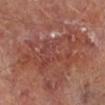Impression: Captured during whole-body skin photography for melanoma surveillance; the lesion was not biopsied. Image and clinical context: About 11 mm across. Cropped from a whole-body photographic skin survey; the tile spans about 15 mm. A male patient aged around 65. The lesion is located on the left lower leg. The total-body-photography lesion software estimated an area of roughly 32 mm², an eccentricity of roughly 0.9, and a symmetry-axis asymmetry near 0.4. It also reported a lesion-detection confidence of about 70/100. Imaged with cross-polarized lighting.